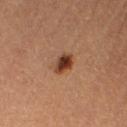biopsy status: catalogued during a skin exam; not biopsied
image-analysis metrics: an automated nevus-likeness rating near 100 out of 100 and lesion-presence confidence of about 100/100
subject: female, roughly 30 years of age
image source: ~15 mm tile from a whole-body skin photo
tile lighting: cross-polarized
body site: the right thigh